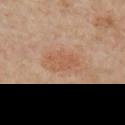<tbp_lesion>
  <biopsy_status>not biopsied; imaged during a skin examination</biopsy_status>
  <automated_metrics>
    <cielab_L>57</cielab_L>
    <cielab_a>22</cielab_a>
    <cielab_b>33</cielab_b>
    <vs_skin_darker_L>6.0</vs_skin_darker_L>
    <vs_skin_contrast_norm>5.0</vs_skin_contrast_norm>
    <border_irregularity_0_10>3.0</border_irregularity_0_10>
    <color_variation_0_10>2.0</color_variation_0_10>
    <peripheral_color_asymmetry>1.0</peripheral_color_asymmetry>
  </automated_metrics>
  <lighting>white-light</lighting>
  <patient>
    <sex>male</sex>
    <age_approx>60</age_approx>
  </patient>
  <image>
    <source>total-body photography crop</source>
    <field_of_view_mm>15</field_of_view_mm>
  </image>
  <site>front of the torso</site>
</tbp_lesion>